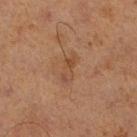Impression: This lesion was catalogued during total-body skin photography and was not selected for biopsy. Image and clinical context: Captured under cross-polarized illumination. Approximately 4 mm at its widest. From the right lower leg. A lesion tile, about 15 mm wide, cut from a 3D total-body photograph. A male subject approximately 70 years of age. Automated image analysis of the tile measured a mean CIELAB color near L≈38 a*≈17 b*≈27, about 5 CIELAB-L* units darker than the surrounding skin, and a normalized border contrast of about 5. The analysis additionally found a color-variation rating of about 3.5/10. It also reported a lesion-detection confidence of about 100/100.